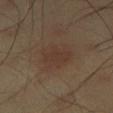follow-up: catalogued during a skin exam; not biopsied
image source: 15 mm crop, total-body photography
diameter: ~5 mm (longest diameter)
anatomic site: the left thigh
patient: male, aged 43 to 47
automated metrics: an average lesion color of about L≈35 a*≈15 b*≈23 (CIELAB) and a lesion-to-skin contrast of about 5 (normalized; higher = more distinct); a nevus-likeness score of about 55/100 and lesion-presence confidence of about 100/100
illumination: cross-polarized illumination